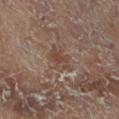workup — imaged on a skin check; not biopsied | lighting — cross-polarized illumination | patient — female, aged 78–82 | image source — 15 mm crop, total-body photography | automated metrics — an area of roughly 7 mm²; a lesion color around L≈39 a*≈15 b*≈22 in CIELAB, about 6 CIELAB-L* units darker than the surrounding skin, and a lesion-to-skin contrast of about 5.5 (normalized; higher = more distinct); a classifier nevus-likeness of about 0/100 and a detector confidence of about 60 out of 100 that the crop contains a lesion | location — the right leg.